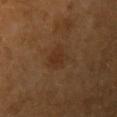workup = catalogued during a skin exam; not biopsied
acquisition = total-body-photography crop, ~15 mm field of view
patient = female, aged 48 to 52
TBP lesion metrics = an area of roughly 5 mm², an eccentricity of roughly 0.55, and two-axis asymmetry of about 0.2; a nevus-likeness score of about 55/100 and a detector confidence of about 100 out of 100 that the crop contains a lesion
body site = the left upper arm